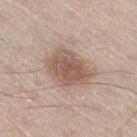biopsy status = imaged on a skin check; not biopsied | subject = male, about 60 years old | size = about 5.5 mm | lighting = white-light | site = the right thigh | image source = ~15 mm tile from a whole-body skin photo.